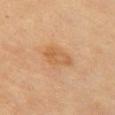Clinical impression: The lesion was tiled from a total-body skin photograph and was not biopsied. Clinical summary: A female patient approximately 80 years of age. From the chest. A 15 mm close-up extracted from a 3D total-body photography capture.